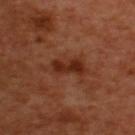This image is a 15 mm lesion crop taken from a total-body photograph. From the back. The subject is a male aged 48–52. About 6 mm across. Imaged with cross-polarized lighting.